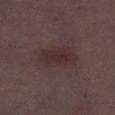The patient is a female aged 28 to 32. The tile uses white-light illumination. Approximately 5 mm at its widest. A lesion tile, about 15 mm wide, cut from a 3D total-body photograph. The lesion is on the left thigh.